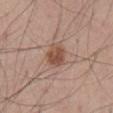Q: Is there a histopathology result?
A: catalogued during a skin exam; not biopsied